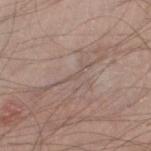The lesion-visualizer software estimated a within-lesion color-variation index near 0/10 and a peripheral color-asymmetry measure near 0. On the left lower leg. A roughly 15 mm field-of-view crop from a total-body skin photograph. Imaged with white-light lighting. Measured at roughly 2 mm in maximum diameter. A male patient in their mid- to late 30s.